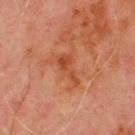The lesion was photographed on a routine skin check and not biopsied; there is no pathology result.
A male patient, approximately 70 years of age.
Captured under cross-polarized illumination.
A lesion tile, about 15 mm wide, cut from a 3D total-body photograph.
The lesion is on the chest.
Measured at roughly 4 mm in maximum diameter.